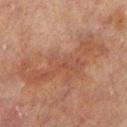Clinical impression:
This lesion was catalogued during total-body skin photography and was not selected for biopsy.
Context:
A male subject, roughly 70 years of age. A 15 mm crop from a total-body photograph taken for skin-cancer surveillance. The total-body-photography lesion software estimated a color-variation rating of about 3.5/10 and a peripheral color-asymmetry measure near 1. Imaged with cross-polarized lighting. The lesion is on the left lower leg.